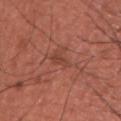This lesion was catalogued during total-body skin photography and was not selected for biopsy. A 15 mm crop from a total-body photograph taken for skin-cancer surveillance. A male subject aged 33 to 37. Located on the chest.